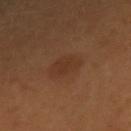The lesion was tiled from a total-body skin photograph and was not biopsied.
A female subject, aged 48–52.
Captured under cross-polarized illumination.
This image is a 15 mm lesion crop taken from a total-body photograph.